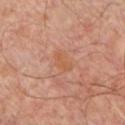– notes · catalogued during a skin exam; not biopsied
– patient · male, aged 53 to 57
– automated lesion analysis · a lesion area of about 4 mm²; roughly 5 lightness units darker than nearby skin; a border-irregularity rating of about 3/10, a color-variation rating of about 2/10, and radial color variation of about 1; an automated nevus-likeness rating near 0 out of 100 and a detector confidence of about 100 out of 100 that the crop contains a lesion
– location · the front of the torso
– image · total-body-photography crop, ~15 mm field of view